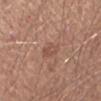Recorded during total-body skin imaging; not selected for excision or biopsy.
About 2.5 mm across.
The patient is a male roughly 40 years of age.
Automated tile analysis of the lesion measured an area of roughly 3.5 mm² and a shape eccentricity near 0.75. The analysis additionally found a border-irregularity index near 2/10 and a within-lesion color-variation index near 2/10. The analysis additionally found a nevus-likeness score of about 0/100.
From the left forearm.
Imaged with white-light lighting.
This image is a 15 mm lesion crop taken from a total-body photograph.